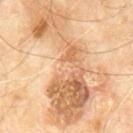Impression:
No biopsy was performed on this lesion — it was imaged during a full skin examination and was not determined to be concerning.
Context:
The subject is a male approximately 60 years of age. On the chest. Cropped from a total-body skin-imaging series; the visible field is about 15 mm. Automated tile analysis of the lesion measured a border-irregularity index near 10/10 and radial color variation of about 3. Longest diameter approximately 15 mm. Captured under cross-polarized illumination.